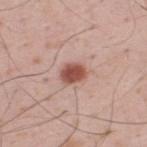<tbp_lesion>
  <biopsy_status>not biopsied; imaged during a skin examination</biopsy_status>
  <site>upper back</site>
  <patient>
    <sex>male</sex>
    <age_approx>35</age_approx>
  </patient>
  <image>
    <source>total-body photography crop</source>
    <field_of_view_mm>15</field_of_view_mm>
  </image>
  <lesion_size>
    <long_diameter_mm_approx>3.0</long_diameter_mm_approx>
  </lesion_size>
</tbp_lesion>